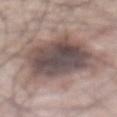  biopsy_status: not biopsied; imaged during a skin examination
  patient:
    sex: male
    age_approx: 65
  lighting: white-light
  site: front of the torso
  lesion_size:
    long_diameter_mm_approx: 9.5
  image:
    source: total-body photography crop
    field_of_view_mm: 15